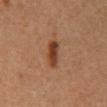The lesion was photographed on a routine skin check and not biopsied; there is no pathology result. This image is a 15 mm lesion crop taken from a total-body photograph. A male patient approximately 60 years of age. The lesion is located on the mid back.